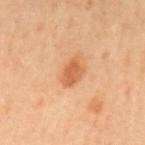Findings:
– workup — imaged on a skin check; not biopsied
– lesion diameter — ~3.5 mm (longest diameter)
– image source — 15 mm crop, total-body photography
– patient — male, aged 63 to 67
– automated metrics — a border-irregularity index near 2/10, a within-lesion color-variation index near 2.5/10, and peripheral color asymmetry of about 1; a classifier nevus-likeness of about 55/100
– lighting — cross-polarized illumination
– location — the mid back